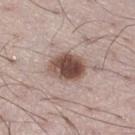workup: imaged on a skin check; not biopsied
location: the right thigh
subject: male, aged 48 to 52
size: ~4.5 mm (longest diameter)
illumination: white-light illumination
automated metrics: a footprint of about 12 mm², an eccentricity of roughly 0.65, and a shape-asymmetry score of about 0.15 (0 = symmetric); a color-variation rating of about 7/10 and radial color variation of about 2.5
image source: 15 mm crop, total-body photography